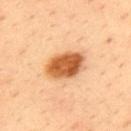biopsy status=imaged on a skin check; not biopsied | illumination=cross-polarized illumination | body site=the upper back | lesion diameter=≈4.5 mm | subject=male, in their mid- to late 30s | automated lesion analysis=a lesion area of about 12 mm², a shape eccentricity near 0.75, and two-axis asymmetry of about 0.15 | imaging modality=total-body-photography crop, ~15 mm field of view.